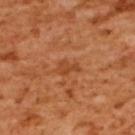- size — about 3 mm
- anatomic site — the upper back
- acquisition — 15 mm crop, total-body photography
- TBP lesion metrics — a lesion area of about 3.5 mm², an outline eccentricity of about 0.8 (0 = round, 1 = elongated), and a symmetry-axis asymmetry near 0.4; an average lesion color of about L≈47 a*≈28 b*≈41 (CIELAB), a lesion–skin lightness drop of about 8, and a normalized border contrast of about 6; an automated nevus-likeness rating near 0 out of 100 and a detector confidence of about 100 out of 100 that the crop contains a lesion
- subject — female, about 55 years old